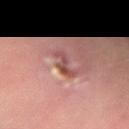Notes:
• workup: catalogued during a skin exam; not biopsied
• location: the left forearm
• diameter: ≈3 mm
• patient: male, aged 53–57
• acquisition: total-body-photography crop, ~15 mm field of view
• automated metrics: an automated nevus-likeness rating near 0 out of 100